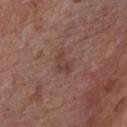Assessment:
Recorded during total-body skin imaging; not selected for excision or biopsy.
Acquisition and patient details:
This is a white-light tile. An algorithmic analysis of the crop reported a lesion color around L≈41 a*≈19 b*≈24 in CIELAB, roughly 6 lightness units darker than nearby skin, and a lesion-to-skin contrast of about 5.5 (normalized; higher = more distinct). It also reported a nevus-likeness score of about 0/100 and lesion-presence confidence of about 100/100. From the chest. About 2.5 mm across. This image is a 15 mm lesion crop taken from a total-body photograph. A male patient aged approximately 60.